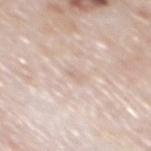Recorded during total-body skin imaging; not selected for excision or biopsy.
About 1.5 mm across.
A region of skin cropped from a whole-body photographic capture, roughly 15 mm wide.
A male patient in their 80s.
On the mid back.